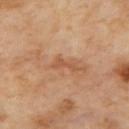Impression:
Captured during whole-body skin photography for melanoma surveillance; the lesion was not biopsied.
Background:
The lesion is located on the back. The recorded lesion diameter is about 2.5 mm. Automated image analysis of the tile measured a lesion-detection confidence of about 100/100. This is a cross-polarized tile. A lesion tile, about 15 mm wide, cut from a 3D total-body photograph.